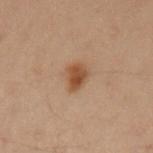Assessment: Part of a total-body skin-imaging series; this lesion was reviewed on a skin check and was not flagged for biopsy. Context: Located on the left upper arm. A female patient aged approximately 40. Cropped from a whole-body photographic skin survey; the tile spans about 15 mm.